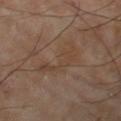Q: Was a biopsy performed?
A: imaged on a skin check; not biopsied